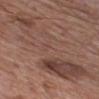workup: imaged on a skin check; not biopsied | image-analysis metrics: a footprint of about 49 mm² and a shape eccentricity near 0.95; a lesion color around L≈45 a*≈18 b*≈24 in CIELAB, about 6 CIELAB-L* units darker than the surrounding skin, and a normalized border contrast of about 5; a nevus-likeness score of about 0/100 and a lesion-detection confidence of about 90/100 | patient: male, about 65 years old | acquisition: total-body-photography crop, ~15 mm field of view | anatomic site: the chest | lesion size: ≈14.5 mm | lighting: white-light.